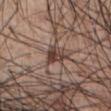The lesion was photographed on a routine skin check and not biopsied; there is no pathology result. Located on the abdomen. A 15 mm close-up tile from a total-body photography series done for melanoma screening. The subject is a male roughly 55 years of age.